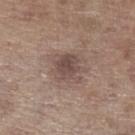notes = catalogued during a skin exam; not biopsied
lesion diameter = ≈3.5 mm
body site = the left lower leg
patient = male, about 70 years old
acquisition = ~15 mm crop, total-body skin-cancer survey